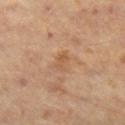Findings:
• follow-up: total-body-photography surveillance lesion; no biopsy
• image source: ~15 mm tile from a whole-body skin photo
• patient: male, approximately 60 years of age
• lighting: cross-polarized
• location: the left thigh
• automated lesion analysis: a mean CIELAB color near L≈51 a*≈18 b*≈33, roughly 6 lightness units darker than nearby skin, and a normalized lesion–skin contrast near 5; border irregularity of about 1.5 on a 0–10 scale, a within-lesion color-variation index near 2/10, and a peripheral color-asymmetry measure near 1